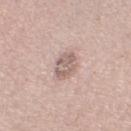Q: Was this lesion biopsied?
A: no biopsy performed (imaged during a skin exam)
Q: How large is the lesion?
A: ~3.5 mm (longest diameter)
Q: Illumination type?
A: white-light
Q: Who is the patient?
A: male, aged 63–67
Q: What kind of image is this?
A: ~15 mm tile from a whole-body skin photo
Q: Lesion location?
A: the left lower leg